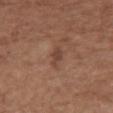Q: Was this lesion biopsied?
A: imaged on a skin check; not biopsied
Q: What is the imaging modality?
A: total-body-photography crop, ~15 mm field of view
Q: Where on the body is the lesion?
A: the chest
Q: What are the patient's age and sex?
A: female, aged approximately 75
Q: What lighting was used for the tile?
A: white-light illumination